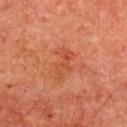imaging modality — ~15 mm crop, total-body skin-cancer survey
subject — male, about 65 years old
lighting — cross-polarized
body site — the chest
lesion size — ≈4 mm